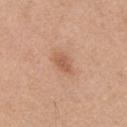The lesion was photographed on a routine skin check and not biopsied; there is no pathology result. This is a white-light tile. A male patient roughly 70 years of age. Longest diameter approximately 3 mm. A close-up tile cropped from a whole-body skin photograph, about 15 mm across. Located on the chest.